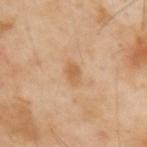| key | value |
|---|---|
| biopsy status | no biopsy performed (imaged during a skin exam) |
| patient | male, aged 53–57 |
| imaging modality | total-body-photography crop, ~15 mm field of view |
| anatomic site | the back |